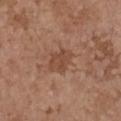Findings:
• body site — the chest
• acquisition — ~15 mm crop, total-body skin-cancer survey
• patient — female, aged 63–67